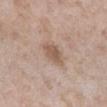Imaged during a routine full-body skin examination; the lesion was not biopsied and no histopathology is available.
This image is a 15 mm lesion crop taken from a total-body photograph.
The lesion's longest dimension is about 3 mm.
On the left lower leg.
Captured under white-light illumination.
The patient is a female approximately 55 years of age.